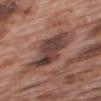anatomic site = the upper back; lesion diameter = ≈6 mm; tile lighting = white-light; subject = male, in their 70s; image source = ~15 mm tile from a whole-body skin photo.